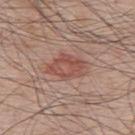notes — no biopsy performed (imaged during a skin exam)
subject — male, aged around 65
acquisition — ~15 mm tile from a whole-body skin photo
body site — the upper back
TBP lesion metrics — a footprint of about 10 mm², an eccentricity of roughly 0.8, and a shape-asymmetry score of about 0.2 (0 = symmetric); border irregularity of about 2 on a 0–10 scale, a color-variation rating of about 4/10, and radial color variation of about 1.5